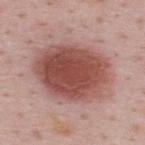The lesion was tiled from a total-body skin photograph and was not biopsied.
From the upper back.
Captured under white-light illumination.
A region of skin cropped from a whole-body photographic capture, roughly 15 mm wide.
An algorithmic analysis of the crop reported an average lesion color of about L≈49 a*≈25 b*≈24 (CIELAB), about 15 CIELAB-L* units darker than the surrounding skin, and a normalized border contrast of about 10.5. The software also gave a border-irregularity rating of about 1.5/10, internal color variation of about 5 on a 0–10 scale, and peripheral color asymmetry of about 1.5. The software also gave an automated nevus-likeness rating near 100 out of 100 and lesion-presence confidence of about 100/100.
A male patient aged 48–52.
Measured at roughly 8 mm in maximum diameter.